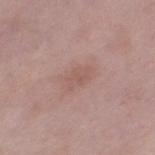workup: imaged on a skin check; not biopsied.